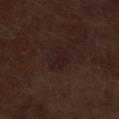Case summary:
– follow-up · catalogued during a skin exam; not biopsied
– size · about 3 mm
– tile lighting · white-light illumination
– location · the left lower leg
– imaging modality · total-body-photography crop, ~15 mm field of view
– patient · male, aged 68–72